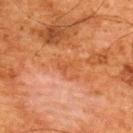follow-up = imaged on a skin check; not biopsied
size = ≈3 mm
acquisition = 15 mm crop, total-body photography
subject = male, in their mid- to late 60s
automated lesion analysis = border irregularity of about 5 on a 0–10 scale, a color-variation rating of about 1/10, and peripheral color asymmetry of about 0
location = the upper back
lighting = cross-polarized